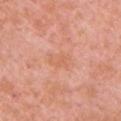{
  "biopsy_status": "not biopsied; imaged during a skin examination",
  "automated_metrics": {
    "area_mm2_approx": 4.5,
    "eccentricity": 0.8,
    "shape_asymmetry": 0.25,
    "vs_skin_darker_L": 5.0,
    "vs_skin_contrast_norm": 4.5,
    "color_variation_0_10": 1.5,
    "peripheral_color_asymmetry": 0.5,
    "lesion_detection_confidence_0_100": 100
  },
  "patient": {
    "sex": "female",
    "age_approx": 40
  },
  "site": "chest",
  "lighting": "white-light",
  "lesion_size": {
    "long_diameter_mm_approx": 3.0
  },
  "image": {
    "source": "total-body photography crop",
    "field_of_view_mm": 15
  }
}